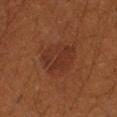follow-up: imaged on a skin check; not biopsied
imaging modality: total-body-photography crop, ~15 mm field of view
size: ≈5 mm
TBP lesion metrics: a border-irregularity rating of about 3/10 and a within-lesion color-variation index near 3.5/10; a nevus-likeness score of about 45/100 and a lesion-detection confidence of about 100/100
subject: male, roughly 55 years of age
location: the left forearm
lighting: cross-polarized